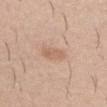{"automated_metrics": {"border_irregularity_0_10": 2.5, "color_variation_0_10": 1.5, "peripheral_color_asymmetry": 0.5}, "lighting": "white-light", "image": {"source": "total-body photography crop", "field_of_view_mm": 15}, "site": "left thigh", "patient": {"sex": "male", "age_approx": 40}}